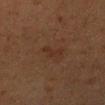notes=catalogued during a skin exam; not biopsied.